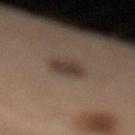Findings:
– follow-up: catalogued during a skin exam; not biopsied
– diameter: ~3.5 mm (longest diameter)
– tile lighting: cross-polarized
– location: the left leg
– TBP lesion metrics: an average lesion color of about L≈37 a*≈13 b*≈21 (CIELAB), about 9 CIELAB-L* units darker than the surrounding skin, and a normalized lesion–skin contrast near 8; a nevus-likeness score of about 90/100
– acquisition: ~15 mm crop, total-body skin-cancer survey
– patient: male, about 50 years old